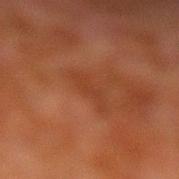No biopsy was performed on this lesion — it was imaged during a full skin examination and was not determined to be concerning. Captured under cross-polarized illumination. A close-up tile cropped from a whole-body skin photograph, about 15 mm across. A male patient, approximately 80 years of age. Automated image analysis of the tile measured an average lesion color of about L≈31 a*≈22 b*≈28 (CIELAB), about 4 CIELAB-L* units darker than the surrounding skin, and a normalized border contrast of about 4.5. The analysis additionally found a peripheral color-asymmetry measure near 0. Measured at roughly 4.5 mm in maximum diameter. The lesion is located on the leg.